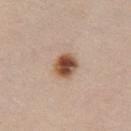About 3 mm across. A female patient, aged 43–47. On the chest. A region of skin cropped from a whole-body photographic capture, roughly 15 mm wide.